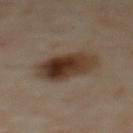Assessment:
The lesion was photographed on a routine skin check and not biopsied; there is no pathology result.
Context:
Imaged with cross-polarized lighting. From the upper back. A region of skin cropped from a whole-body photographic capture, roughly 15 mm wide. The subject is a female aged approximately 60.